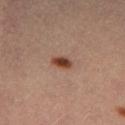notes = total-body-photography surveillance lesion; no biopsy
imaging modality = ~15 mm crop, total-body skin-cancer survey
lesion diameter = ≈2.5 mm
subject = female, roughly 45 years of age
tile lighting = cross-polarized
body site = the left thigh